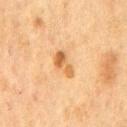Clinical impression: Captured during whole-body skin photography for melanoma surveillance; the lesion was not biopsied.